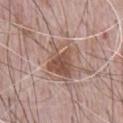Imaged during a routine full-body skin examination; the lesion was not biopsied and no histopathology is available.
A close-up tile cropped from a whole-body skin photograph, about 15 mm across.
Located on the chest.
A male subject about 70 years old.
The lesion's longest dimension is about 4.5 mm.